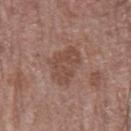{"biopsy_status": "not biopsied; imaged during a skin examination", "patient": {"sex": "male", "age_approx": 75}, "image": {"source": "total-body photography crop", "field_of_view_mm": 15}, "automated_metrics": {"eccentricity": 0.5, "shape_asymmetry": 0.2}, "site": "arm", "lighting": "white-light", "lesion_size": {"long_diameter_mm_approx": 4.5}}